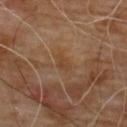Imaged during a routine full-body skin examination; the lesion was not biopsied and no histopathology is available. Located on the upper back. Imaged with cross-polarized lighting. The patient is a male approximately 65 years of age. The lesion-visualizer software estimated a mean CIELAB color near L≈40 a*≈18 b*≈31, roughly 5 lightness units darker than nearby skin, and a normalized border contrast of about 5.5. This image is a 15 mm lesion crop taken from a total-body photograph.